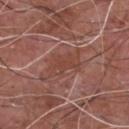Q: Is there a histopathology result?
A: imaged on a skin check; not biopsied
Q: What is the anatomic site?
A: the chest
Q: Who is the patient?
A: male, aged 63 to 67
Q: What kind of image is this?
A: ~15 mm crop, total-body skin-cancer survey